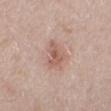* workup · imaged on a skin check; not biopsied
* lesion size · ≈3.5 mm
* patient · female, aged approximately 30
* location · the mid back
* lighting · white-light
* acquisition · ~15 mm tile from a whole-body skin photo